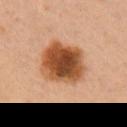Notes:
• workup · total-body-photography surveillance lesion; no biopsy
• subject · female, approximately 30 years of age
• body site · the right upper arm
• tile lighting · cross-polarized
• imaging modality · 15 mm crop, total-body photography
• automated metrics · a lesion area of about 23 mm², an outline eccentricity of about 0.45 (0 = round, 1 = elongated), and a shape-asymmetry score of about 0.15 (0 = symmetric); a nevus-likeness score of about 90/100 and lesion-presence confidence of about 100/100
• lesion diameter · about 5.5 mm